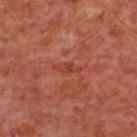Clinical summary:
An algorithmic analysis of the crop reported a mean CIELAB color near L≈37 a*≈29 b*≈31, about 6 CIELAB-L* units darker than the surrounding skin, and a normalized border contrast of about 6. It also reported a within-lesion color-variation index near 0/10 and peripheral color asymmetry of about 0. Located on the chest. A male patient aged around 60. Captured under cross-polarized illumination. A 15 mm close-up extracted from a 3D total-body photography capture.